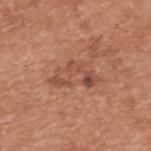No biopsy was performed on this lesion — it was imaged during a full skin examination and was not determined to be concerning.
The patient is a male in their mid- to late 50s.
Located on the upper back.
A close-up tile cropped from a whole-body skin photograph, about 15 mm across.
The lesion's longest dimension is about 5.5 mm.